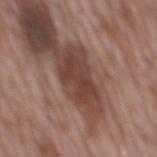notes = catalogued during a skin exam; not biopsied | TBP lesion metrics = a footprint of about 11 mm², a shape eccentricity near 0.8, and a symmetry-axis asymmetry near 0.35; about 10 CIELAB-L* units darker than the surrounding skin and a normalized lesion–skin contrast near 8.5; a border-irregularity rating of about 4.5/10 and a within-lesion color-variation index near 3/10; a nevus-likeness score of about 10/100 and a detector confidence of about 85 out of 100 that the crop contains a lesion | lesion size = about 5 mm | subject = male, aged 68–72 | image source = ~15 mm tile from a whole-body skin photo | illumination = white-light illumination | location = the mid back.